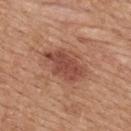| feature | finding |
|---|---|
| biopsy status | no biopsy performed (imaged during a skin exam) |
| imaging modality | 15 mm crop, total-body photography |
| location | the back |
| diameter | ~6 mm (longest diameter) |
| lighting | white-light |
| subject | female, aged approximately 45 |